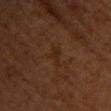Imaged during a routine full-body skin examination; the lesion was not biopsied and no histopathology is available. Automated tile analysis of the lesion measured an outline eccentricity of about 0.8 (0 = round, 1 = elongated) and a symmetry-axis asymmetry near 0.45. It also reported a mean CIELAB color near L≈20 a*≈16 b*≈24, about 4 CIELAB-L* units darker than the surrounding skin, and a lesion-to-skin contrast of about 5.5 (normalized; higher = more distinct). The analysis additionally found border irregularity of about 5 on a 0–10 scale, a within-lesion color-variation index near 1/10, and a peripheral color-asymmetry measure near 0.5. A female subject, aged 48–52. This is a cross-polarized tile. The lesion is located on the front of the torso. Approximately 3 mm at its widest. A lesion tile, about 15 mm wide, cut from a 3D total-body photograph.